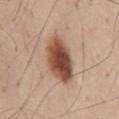Notes:
- workup: total-body-photography surveillance lesion; no biopsy
- TBP lesion metrics: a within-lesion color-variation index near 8/10
- diameter: ~6 mm (longest diameter)
- acquisition: 15 mm crop, total-body photography
- lighting: white-light illumination
- patient: male, aged 58–62
- body site: the mid back